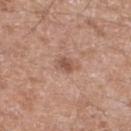Recorded during total-body skin imaging; not selected for excision or biopsy. The lesion is located on the right lower leg. This image is a 15 mm lesion crop taken from a total-body photograph. The recorded lesion diameter is about 2.5 mm. A male patient aged 58–62. The lesion-visualizer software estimated a shape-asymmetry score of about 0.35 (0 = symmetric). And it measured radial color variation of about 0.5. The analysis additionally found a classifier nevus-likeness of about 55/100 and a detector confidence of about 100 out of 100 that the crop contains a lesion.